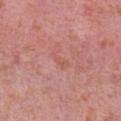The lesion was photographed on a routine skin check and not biopsied; there is no pathology result. A female subject, in their 40s. From the left forearm. A 15 mm crop from a total-body photograph taken for skin-cancer surveillance.